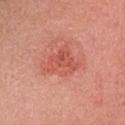Recorded during total-body skin imaging; not selected for excision or biopsy. A roughly 15 mm field-of-view crop from a total-body skin photograph. About 4.5 mm across. From the head or neck. Captured under white-light illumination. A female patient, about 50 years old.